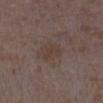This lesion was catalogued during total-body skin photography and was not selected for biopsy.
Longest diameter approximately 3 mm.
The lesion is located on the right lower leg.
Captured under white-light illumination.
Automated image analysis of the tile measured a footprint of about 6 mm² and an eccentricity of roughly 0.65. The analysis additionally found a border-irregularity rating of about 2/10, internal color variation of about 1.5 on a 0–10 scale, and radial color variation of about 0.5.
A 15 mm crop from a total-body photograph taken for skin-cancer surveillance.
The patient is a female aged around 35.